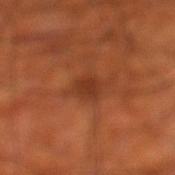Recorded during total-body skin imaging; not selected for excision or biopsy.
Automated image analysis of the tile measured roughly 8 lightness units darker than nearby skin and a normalized border contrast of about 7. The software also gave a classifier nevus-likeness of about 0/100.
Located on the left lower leg.
The subject is a male aged approximately 70.
A 15 mm close-up extracted from a 3D total-body photography capture.
Longest diameter approximately 2.5 mm.
This is a cross-polarized tile.